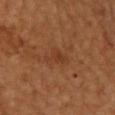Findings:
• notes — total-body-photography surveillance lesion; no biopsy
• patient — male, aged 63 to 67
• imaging modality — ~15 mm crop, total-body skin-cancer survey
• body site — the upper back
• lighting — cross-polarized illumination
• diameter — ≈3 mm